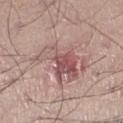No biopsy was performed on this lesion — it was imaged during a full skin examination and was not determined to be concerning. The patient is a male roughly 55 years of age. A lesion tile, about 15 mm wide, cut from a 3D total-body photograph. Longest diameter approximately 6.5 mm. The tile uses white-light illumination. The lesion-visualizer software estimated an area of roughly 16 mm² and an eccentricity of roughly 0.8. The software also gave roughly 10 lightness units darker than nearby skin and a normalized border contrast of about 7.